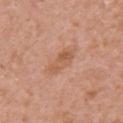workup: imaged on a skin check; not biopsied | anatomic site: the right upper arm | tile lighting: white-light illumination | patient: female, about 40 years old | lesion diameter: ≈5 mm | imaging modality: ~15 mm tile from a whole-body skin photo | image-analysis metrics: a border-irregularity rating of about 3/10, a within-lesion color-variation index near 3.5/10, and a peripheral color-asymmetry measure near 1.5; an automated nevus-likeness rating near 0 out of 100 and a detector confidence of about 100 out of 100 that the crop contains a lesion.